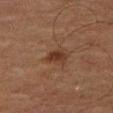  biopsy_status: not biopsied; imaged during a skin examination
  patient:
    sex: male
    age_approx: 75
  image:
    source: total-body photography crop
    field_of_view_mm: 15
  lesion_size:
    long_diameter_mm_approx: 2.5
  site: right thigh